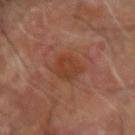The lesion was tiled from a total-body skin photograph and was not biopsied. This image is a 15 mm lesion crop taken from a total-body photograph. Imaged with cross-polarized lighting. From the right forearm. A male patient, about 70 years old. Approximately 4 mm at its widest.